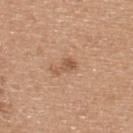biopsy status — imaged on a skin check; not biopsied | patient — male, aged 38–42 | image — total-body-photography crop, ~15 mm field of view | automated metrics — a shape eccentricity near 0.85 and two-axis asymmetry of about 0.5; roughly 9 lightness units darker than nearby skin and a lesion-to-skin contrast of about 6.5 (normalized; higher = more distinct); a border-irregularity index near 5/10, a color-variation rating of about 2.5/10, and peripheral color asymmetry of about 1 | lighting — white-light | location — the upper back | size — about 3 mm.